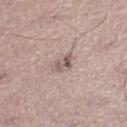Impression: This lesion was catalogued during total-body skin photography and was not selected for biopsy. Context: Measured at roughly 3 mm in maximum diameter. A male subject, aged around 45. This is a white-light tile. A close-up tile cropped from a whole-body skin photograph, about 15 mm across. From the leg. Automated tile analysis of the lesion measured a lesion-detection confidence of about 70/100.